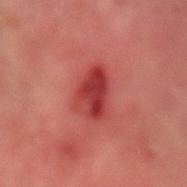Impression: Recorded during total-body skin imaging; not selected for excision or biopsy. Acquisition and patient details: A roughly 15 mm field-of-view crop from a total-body skin photograph. A male patient, in their mid- to late 60s. The lesion is on the right forearm. Automated tile analysis of the lesion measured an average lesion color of about L≈42 a*≈39 b*≈29 (CIELAB) and a lesion-to-skin contrast of about 9 (normalized; higher = more distinct). It also reported border irregularity of about 3 on a 0–10 scale, a within-lesion color-variation index near 6/10, and peripheral color asymmetry of about 2. The tile uses cross-polarized illumination.